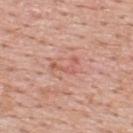No biopsy was performed on this lesion — it was imaged during a full skin examination and was not determined to be concerning. Automated tile analysis of the lesion measured a footprint of about 3 mm² and a symmetry-axis asymmetry near 0.8. And it measured a border-irregularity rating of about 9.5/10, a color-variation rating of about 0/10, and peripheral color asymmetry of about 0. The analysis additionally found a nevus-likeness score of about 0/100. From the back. About 3 mm across. A close-up tile cropped from a whole-body skin photograph, about 15 mm across. Captured under white-light illumination. A male subject aged around 55.